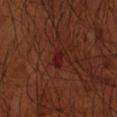Findings:
* follow-up · imaged on a skin check; not biopsied
* subject · male, in their 50s
* acquisition · 15 mm crop, total-body photography
* anatomic site · the left arm
* automated lesion analysis · an average lesion color of about L≈21 a*≈28 b*≈24 (CIELAB), a lesion–skin lightness drop of about 6, and a normalized border contrast of about 7; a border-irregularity index near 2.5/10, internal color variation of about 1.5 on a 0–10 scale, and a peripheral color-asymmetry measure near 0.5; an automated nevus-likeness rating near 0 out of 100 and lesion-presence confidence of about 100/100
* tile lighting · cross-polarized
* diameter · about 2.5 mm